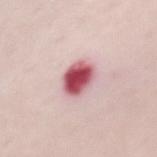Q: Was a biopsy performed?
A: catalogued during a skin exam; not biopsied
Q: Where on the body is the lesion?
A: the chest
Q: How large is the lesion?
A: ≈3.5 mm
Q: What is the imaging modality?
A: ~15 mm crop, total-body skin-cancer survey
Q: What did automated image analysis measure?
A: roughly 22 lightness units darker than nearby skin and a normalized lesion–skin contrast near 13; border irregularity of about 1.5 on a 0–10 scale and radial color variation of about 2
Q: Illumination type?
A: white-light
Q: What are the patient's age and sex?
A: female, about 65 years old